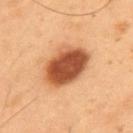Recorded during total-body skin imaging; not selected for excision or biopsy. Measured at roughly 6 mm in maximum diameter. The lesion is located on the upper back. Imaged with cross-polarized lighting. A male patient aged around 55. A roughly 15 mm field-of-view crop from a total-body skin photograph.